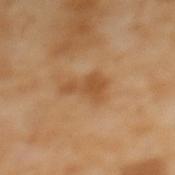<case>
<biopsy_status>not biopsied; imaged during a skin examination</biopsy_status>
<image>
  <source>total-body photography crop</source>
  <field_of_view_mm>15</field_of_view_mm>
</image>
<lighting>cross-polarized</lighting>
<patient>
  <sex>female</sex>
  <age_approx>55</age_approx>
</patient>
<lesion_size>
  <long_diameter_mm_approx>4.0</long_diameter_mm_approx>
</lesion_size>
<site>mid back</site>
</case>